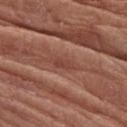Q: Who is the patient?
A: female, aged 73 to 77
Q: What is the lesion's diameter?
A: ~2.5 mm (longest diameter)
Q: Where on the body is the lesion?
A: the left forearm
Q: Illumination type?
A: white-light illumination
Q: How was this image acquired?
A: ~15 mm crop, total-body skin-cancer survey
Q: What did automated image analysis measure?
A: a border-irregularity index near 2.5/10 and a peripheral color-asymmetry measure near 0.5